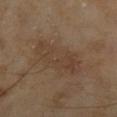Findings:
- biopsy status · catalogued during a skin exam; not biopsied
- site · the leg
- patient · male, aged 63–67
- image · ~15 mm crop, total-body skin-cancer survey